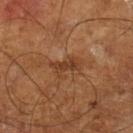follow-up — total-body-photography surveillance lesion; no biopsy
automated lesion analysis — a lesion area of about 6.5 mm², a shape eccentricity near 0.8, and a symmetry-axis asymmetry near 0.35; a classifier nevus-likeness of about 0/100 and a detector confidence of about 95 out of 100 that the crop contains a lesion
acquisition — total-body-photography crop, ~15 mm field of view
subject — male, aged 63 to 67
illumination — cross-polarized illumination
anatomic site — the left lower leg